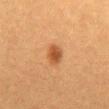Case summary:
- follow-up · no biopsy performed (imaged during a skin exam)
- tile lighting · cross-polarized illumination
- acquisition · ~15 mm tile from a whole-body skin photo
- subject · female, aged 38–42
- anatomic site · the abdomen